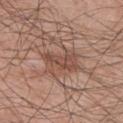Q: Who is the patient?
A: male, approximately 45 years of age
Q: What is the imaging modality?
A: total-body-photography crop, ~15 mm field of view
Q: What is the anatomic site?
A: the left upper arm
Q: How large is the lesion?
A: ~4 mm (longest diameter)
Q: What lighting was used for the tile?
A: white-light